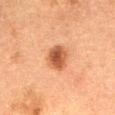The subject is a female aged approximately 50.
Cropped from a total-body skin-imaging series; the visible field is about 15 mm.
Captured under cross-polarized illumination.
From the abdomen.
Automated tile analysis of the lesion measured a classifier nevus-likeness of about 100/100.